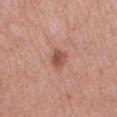biopsy status=catalogued during a skin exam; not biopsied | TBP lesion metrics=a mean CIELAB color near L≈52 a*≈25 b*≈28, about 11 CIELAB-L* units darker than the surrounding skin, and a normalized lesion–skin contrast near 7.5; internal color variation of about 2.5 on a 0–10 scale and peripheral color asymmetry of about 1; a classifier nevus-likeness of about 60/100 and lesion-presence confidence of about 100/100 | image=15 mm crop, total-body photography | body site=the abdomen | lesion diameter=~2.5 mm (longest diameter) | patient=female, in their mid-70s.